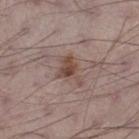A 15 mm close-up tile from a total-body photography series done for melanoma screening.
From the left thigh.
A male subject, aged approximately 70.
About 4 mm across.